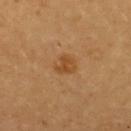| field | value |
|---|---|
| biopsy status | imaged on a skin check; not biopsied |
| image source | ~15 mm tile from a whole-body skin photo |
| lesion diameter | about 2.5 mm |
| location | the upper back |
| patient | female, approximately 60 years of age |